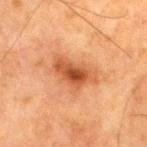site: the right thigh; subject: male, approximately 80 years of age; image: total-body-photography crop, ~15 mm field of view; diameter: ≈5 mm; lighting: cross-polarized.